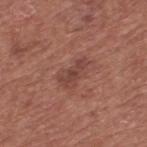Q: Is there a histopathology result?
A: imaged on a skin check; not biopsied
Q: How was this image acquired?
A: total-body-photography crop, ~15 mm field of view
Q: What did automated image analysis measure?
A: a border-irregularity rating of about 4.5/10, a within-lesion color-variation index near 2.5/10, and peripheral color asymmetry of about 1; a nevus-likeness score of about 5/100
Q: Where on the body is the lesion?
A: the upper back
Q: Lesion size?
A: ~4 mm (longest diameter)
Q: What are the patient's age and sex?
A: male, about 75 years old